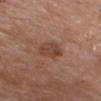{"patient": {"sex": "female", "age_approx": 75}, "site": "chest", "image": {"source": "total-body photography crop", "field_of_view_mm": 15}}